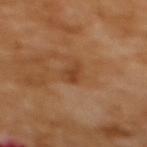Clinical impression:
This lesion was catalogued during total-body skin photography and was not selected for biopsy.
Acquisition and patient details:
The tile uses cross-polarized illumination. From the upper back. Cropped from a total-body skin-imaging series; the visible field is about 15 mm. The patient is a female in their mid- to late 50s.